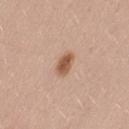* follow-up: total-body-photography surveillance lesion; no biopsy
* illumination: white-light
* subject: female, aged 28–32
* size: ≈3 mm
* acquisition: total-body-photography crop, ~15 mm field of view
* site: the leg